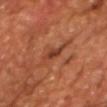Cropped from a whole-body photographic skin survey; the tile spans about 15 mm. The lesion's longest dimension is about 4 mm. A male patient aged around 65. Captured under cross-polarized illumination.